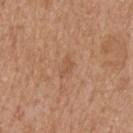Clinical impression:
Captured during whole-body skin photography for melanoma surveillance; the lesion was not biopsied.
Context:
Captured under white-light illumination. A male patient about 60 years old. The lesion-visualizer software estimated an area of roughly 2.5 mm², an outline eccentricity of about 0.85 (0 = round, 1 = elongated), and two-axis asymmetry of about 0.45. It also reported an average lesion color of about L≈54 a*≈21 b*≈33 (CIELAB) and a lesion-to-skin contrast of about 5 (normalized; higher = more distinct). And it measured a border-irregularity index near 4.5/10 and a color-variation rating of about 1/10. The software also gave a classifier nevus-likeness of about 0/100 and a lesion-detection confidence of about 100/100. On the head or neck. This image is a 15 mm lesion crop taken from a total-body photograph.